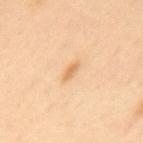Captured during whole-body skin photography for melanoma surveillance; the lesion was not biopsied. A 15 mm close-up extracted from a 3D total-body photography capture. Located on the mid back. Automated tile analysis of the lesion measured a footprint of about 2.5 mm², a shape eccentricity near 0.9, and a shape-asymmetry score of about 0.2 (0 = symmetric). The software also gave a mean CIELAB color near L≈71 a*≈21 b*≈42, about 10 CIELAB-L* units darker than the surrounding skin, and a lesion-to-skin contrast of about 6.5 (normalized; higher = more distinct). The analysis additionally found internal color variation of about 0 on a 0–10 scale. A male patient, aged 63 to 67.